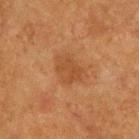Acquisition and patient details:
A male subject, in their mid-70s. This is a cross-polarized tile. The total-body-photography lesion software estimated an area of roughly 7.5 mm², an eccentricity of roughly 0.65, and a symmetry-axis asymmetry near 0.3. And it measured an average lesion color of about L≈39 a*≈20 b*≈32 (CIELAB), about 6 CIELAB-L* units darker than the surrounding skin, and a lesion-to-skin contrast of about 5.5 (normalized; higher = more distinct). The software also gave a border-irregularity rating of about 3.5/10, a color-variation rating of about 2.5/10, and radial color variation of about 1. About 4 mm across. A 15 mm crop from a total-body photograph taken for skin-cancer surveillance. From the upper back.